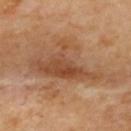Impression:
No biopsy was performed on this lesion — it was imaged during a full skin examination and was not determined to be concerning.
Context:
The lesion is located on the upper back. A 15 mm crop from a total-body photograph taken for skin-cancer surveillance. Longest diameter approximately 11 mm. The total-body-photography lesion software estimated an average lesion color of about L≈52 a*≈21 b*≈34 (CIELAB), about 10 CIELAB-L* units darker than the surrounding skin, and a normalized border contrast of about 7.